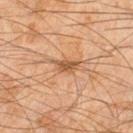workup — catalogued during a skin exam; not biopsied | image source — ~15 mm crop, total-body skin-cancer survey | anatomic site — the right lower leg | illumination — cross-polarized illumination | automated metrics — an area of roughly 4 mm², an eccentricity of roughly 0.8, and two-axis asymmetry of about 0.3; an average lesion color of about L≈44 a*≈18 b*≈31 (CIELAB), roughly 9 lightness units darker than nearby skin, and a lesion-to-skin contrast of about 7.5 (normalized; higher = more distinct); a classifier nevus-likeness of about 5/100 and lesion-presence confidence of about 100/100 | patient — male, aged 43 to 47.